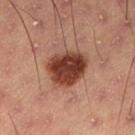The lesion was tiled from a total-body skin photograph and was not biopsied. The lesion is on the abdomen. Approximately 5 mm at its widest. A 15 mm close-up extracted from a 3D total-body photography capture. Automated image analysis of the tile measured an area of roughly 15 mm² and a shape eccentricity near 0.55. The analysis additionally found border irregularity of about 1.5 on a 0–10 scale, a color-variation rating of about 4.5/10, and a peripheral color-asymmetry measure near 1.5. The patient is a male about 55 years old.